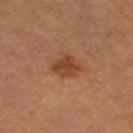Case summary:
• biopsy status — no biopsy performed (imaged during a skin exam)
• lesion size — ~3.5 mm (longest diameter)
• image source — ~15 mm crop, total-body skin-cancer survey
• patient — female, about 60 years old
• tile lighting — cross-polarized illumination
• location — the right thigh
• automated lesion analysis — two-axis asymmetry of about 0.25; a classifier nevus-likeness of about 85/100 and a detector confidence of about 100 out of 100 that the crop contains a lesion